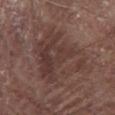Q: Was a biopsy performed?
A: no biopsy performed (imaged during a skin exam)
Q: How was this image acquired?
A: 15 mm crop, total-body photography
Q: Lesion location?
A: the chest
Q: What lighting was used for the tile?
A: white-light
Q: What is the lesion's diameter?
A: ≈9.5 mm
Q: Who is the patient?
A: male, approximately 80 years of age